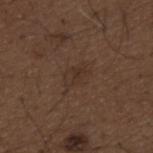Q: Is there a histopathology result?
A: catalogued during a skin exam; not biopsied
Q: What did automated image analysis measure?
A: a footprint of about 6 mm², an outline eccentricity of about 0.85 (0 = round, 1 = elongated), and a symmetry-axis asymmetry near 0.35; a border-irregularity rating of about 4.5/10 and a peripheral color-asymmetry measure near 1
Q: Patient demographics?
A: male, aged approximately 50
Q: How was this image acquired?
A: ~15 mm crop, total-body skin-cancer survey
Q: Lesion location?
A: the mid back
Q: How large is the lesion?
A: ~4 mm (longest diameter)